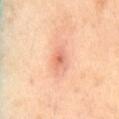biopsy_status: not biopsied; imaged during a skin examination
site: back
image:
  source: total-body photography crop
  field_of_view_mm: 15
automated_metrics:
  shape_asymmetry: 0.3
  cielab_L: 67
  cielab_a: 27
  cielab_b: 34
  vs_skin_contrast_norm: 6.0
  lesion_detection_confidence_0_100: 100
patient:
  sex: male
  age_approx: 55
lighting: cross-polarized
lesion_size:
  long_diameter_mm_approx: 3.0